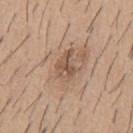notes — total-body-photography surveillance lesion; no biopsy | imaging modality — total-body-photography crop, ~15 mm field of view | location — the mid back | patient — male, aged approximately 60 | size — about 4 mm | tile lighting — white-light illumination.